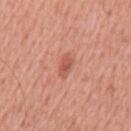workup: imaged on a skin check; not biopsied